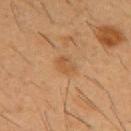Clinical impression: Imaged during a routine full-body skin examination; the lesion was not biopsied and no histopathology is available. Background: Captured under cross-polarized illumination. A male subject, aged 53–57. Cropped from a total-body skin-imaging series; the visible field is about 15 mm. The lesion is located on the chest. Approximately 2.5 mm at its widest.